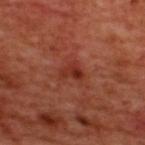No biopsy was performed on this lesion — it was imaged during a full skin examination and was not determined to be concerning.
Located on the upper back.
Cropped from a total-body skin-imaging series; the visible field is about 15 mm.
The patient is a male roughly 50 years of age.